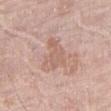Captured during whole-body skin photography for melanoma surveillance; the lesion was not biopsied.
A male patient, aged 78 to 82.
The lesion is located on the right thigh.
A 15 mm close-up extracted from a 3D total-body photography capture.
The lesion-visualizer software estimated an area of roughly 8 mm², an outline eccentricity of about 0.65 (0 = round, 1 = elongated), and two-axis asymmetry of about 0.55.
Approximately 4 mm at its widest.